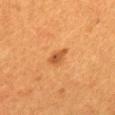{
  "image": {
    "source": "total-body photography crop",
    "field_of_view_mm": 15
  },
  "lighting": "cross-polarized",
  "site": "mid back",
  "lesion_size": {
    "long_diameter_mm_approx": 3.0
  },
  "patient": {
    "sex": "female",
    "age_approx": 55
  }
}